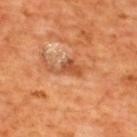Recorded during total-body skin imaging; not selected for excision or biopsy. The subject is a male in their mid- to late 60s. A roughly 15 mm field-of-view crop from a total-body skin photograph. From the upper back. Longest diameter approximately 4.5 mm. Imaged with cross-polarized lighting.